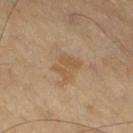Assessment:
The lesion was tiled from a total-body skin photograph and was not biopsied.
Image and clinical context:
A close-up tile cropped from a whole-body skin photograph, about 15 mm across. A male subject, approximately 70 years of age. Imaged with cross-polarized lighting. On the right thigh. Longest diameter approximately 3.5 mm.